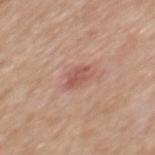Part of a total-body skin-imaging series; this lesion was reviewed on a skin check and was not flagged for biopsy. An algorithmic analysis of the crop reported an eccentricity of roughly 0.7 and a symmetry-axis asymmetry near 0.25. It also reported border irregularity of about 2.5 on a 0–10 scale and a peripheral color-asymmetry measure near 1. The software also gave a nevus-likeness score of about 55/100. Measured at roughly 2.5 mm in maximum diameter. The lesion is located on the back. Cropped from a whole-body photographic skin survey; the tile spans about 15 mm. A male patient aged 58–62. This is a white-light tile.